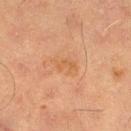No biopsy was performed on this lesion — it was imaged during a full skin examination and was not determined to be concerning.
The lesion is on the left thigh.
Captured under cross-polarized illumination.
A male patient, aged around 65.
A 15 mm close-up tile from a total-body photography series done for melanoma screening.